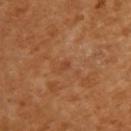Part of a total-body skin-imaging series; this lesion was reviewed on a skin check and was not flagged for biopsy. A 15 mm close-up tile from a total-body photography series done for melanoma screening. A male subject in their 50s. Measured at roughly 1 mm in maximum diameter. The lesion is on the upper back. Imaged with cross-polarized lighting. An algorithmic analysis of the crop reported a lesion area of about 1 mm², an eccentricity of roughly 0.7, and a shape-asymmetry score of about 0.25 (0 = symmetric). The software also gave an average lesion color of about L≈41 a*≈24 b*≈34 (CIELAB), about 6 CIELAB-L* units darker than the surrounding skin, and a lesion-to-skin contrast of about 5 (normalized; higher = more distinct).